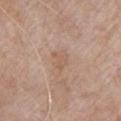Q: Where on the body is the lesion?
A: the chest
Q: Automated lesion metrics?
A: a footprint of about 4 mm², an eccentricity of roughly 0.65, and two-axis asymmetry of about 0.3; a border-irregularity index near 4/10, a color-variation rating of about 1/10, and a peripheral color-asymmetry measure near 0.5
Q: What lighting was used for the tile?
A: white-light
Q: Who is the patient?
A: male, roughly 80 years of age
Q: What kind of image is this?
A: total-body-photography crop, ~15 mm field of view
Q: How large is the lesion?
A: ≈2.5 mm